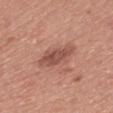Q: Was this lesion biopsied?
A: imaged on a skin check; not biopsied
Q: What are the patient's age and sex?
A: female, approximately 35 years of age
Q: Lesion size?
A: about 5 mm
Q: What is the imaging modality?
A: total-body-photography crop, ~15 mm field of view
Q: Lesion location?
A: the mid back
Q: Automated lesion metrics?
A: a lesion area of about 8.5 mm², a shape eccentricity near 0.9, and a symmetry-axis asymmetry near 0.25; a border-irregularity index near 3.5/10, a within-lesion color-variation index near 3/10, and peripheral color asymmetry of about 1; a nevus-likeness score of about 35/100 and a lesion-detection confidence of about 100/100
Q: How was the tile lit?
A: white-light illumination